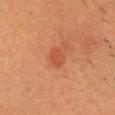Q: Was this lesion biopsied?
A: total-body-photography surveillance lesion; no biopsy
Q: What did automated image analysis measure?
A: an area of roughly 4 mm², an outline eccentricity of about 0.75 (0 = round, 1 = elongated), and a shape-asymmetry score of about 0.25 (0 = symmetric); border irregularity of about 2 on a 0–10 scale, internal color variation of about 1.5 on a 0–10 scale, and peripheral color asymmetry of about 0.5; a lesion-detection confidence of about 100/100
Q: Lesion size?
A: ≈2.5 mm
Q: Patient demographics?
A: male, aged 38–42
Q: What kind of image is this?
A: ~15 mm tile from a whole-body skin photo
Q: What lighting was used for the tile?
A: cross-polarized
Q: Where on the body is the lesion?
A: the head or neck